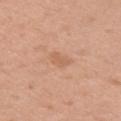Assessment:
The lesion was photographed on a routine skin check and not biopsied; there is no pathology result.
Background:
About 2.5 mm across. A female subject, in their 50s. Automated image analysis of the tile measured an area of roughly 4 mm², a shape eccentricity near 0.75, and two-axis asymmetry of about 0.2. The software also gave about 6 CIELAB-L* units darker than the surrounding skin and a normalized border contrast of about 4.5. And it measured a classifier nevus-likeness of about 0/100 and a lesion-detection confidence of about 100/100. Located on the right upper arm. Cropped from a total-body skin-imaging series; the visible field is about 15 mm.